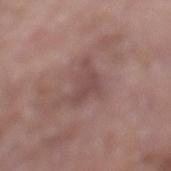<record>
  <site>leg</site>
  <image>
    <source>total-body photography crop</source>
    <field_of_view_mm>15</field_of_view_mm>
  </image>
  <patient>
    <sex>female</sex>
    <age_approx>85+</age_approx>
  </patient>
  <automated_metrics>
    <border_irregularity_0_10>6.0</border_irregularity_0_10>
    <color_variation_0_10>1.5</color_variation_0_10>
    <peripheral_color_asymmetry>0.5</peripheral_color_asymmetry>
  </automated_metrics>
  <lighting>white-light</lighting>
</record>